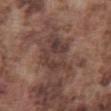Clinical impression:
Recorded during total-body skin imaging; not selected for excision or biopsy.
Background:
Captured under white-light illumination. The patient is a male aged approximately 75. From the abdomen. The lesion-visualizer software estimated a border-irregularity rating of about 5/10, a color-variation rating of about 5/10, and a peripheral color-asymmetry measure near 1.5. It also reported a classifier nevus-likeness of about 0/100 and lesion-presence confidence of about 60/100. Approximately 5.5 mm at its widest. A 15 mm crop from a total-body photograph taken for skin-cancer surveillance.